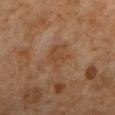Imaged during a routine full-body skin examination; the lesion was not biopsied and no histopathology is available.
Imaged with cross-polarized lighting.
A female subject aged 48 to 52.
A roughly 15 mm field-of-view crop from a total-body skin photograph.
Longest diameter approximately 3 mm.
The lesion is located on the left lower leg.